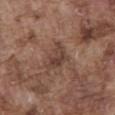Recorded during total-body skin imaging; not selected for excision or biopsy. Cropped from a whole-body photographic skin survey; the tile spans about 15 mm. From the front of the torso. The subject is a male about 75 years old.